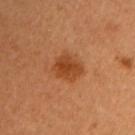Assessment: No biopsy was performed on this lesion — it was imaged during a full skin examination and was not determined to be concerning. Clinical summary: The patient is a female aged around 50. A region of skin cropped from a whole-body photographic capture, roughly 15 mm wide.